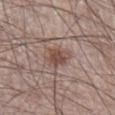{"biopsy_status": "not biopsied; imaged during a skin examination", "automated_metrics": {"area_mm2_approx": 5.5, "eccentricity": 0.45, "shape_asymmetry": 0.25, "cielab_L": 48, "cielab_a": 19, "cielab_b": 23, "vs_skin_darker_L": 10.0, "vs_skin_contrast_norm": 7.5}, "patient": {"sex": "male", "age_approx": 60}, "site": "right lower leg", "image": {"source": "total-body photography crop", "field_of_view_mm": 15}, "lesion_size": {"long_diameter_mm_approx": 3.0}}